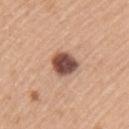Part of a total-body skin-imaging series; this lesion was reviewed on a skin check and was not flagged for biopsy. Imaged with white-light lighting. A roughly 15 mm field-of-view crop from a total-body skin photograph. The patient is a female approximately 55 years of age. The lesion-visualizer software estimated an area of roughly 8 mm², an eccentricity of roughly 0.6, and two-axis asymmetry of about 0.2. The software also gave an average lesion color of about L≈50 a*≈22 b*≈26 (CIELAB), a lesion–skin lightness drop of about 20, and a normalized border contrast of about 13. The software also gave an automated nevus-likeness rating near 75 out of 100 and a detector confidence of about 100 out of 100 that the crop contains a lesion. The lesion is located on the left upper arm. Approximately 3.5 mm at its widest.